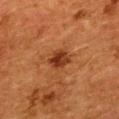follow-up: no biopsy performed (imaged during a skin exam) | acquisition: ~15 mm crop, total-body skin-cancer survey | patient: female, in their 50s | body site: the upper back.